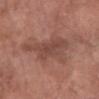biopsy status: no biopsy performed (imaged during a skin exam); image source: 15 mm crop, total-body photography; location: the left forearm; subject: female, aged 68 to 72; lighting: white-light illumination.